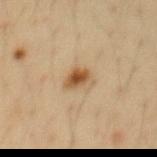Case summary:
- workup: no biopsy performed (imaged during a skin exam)
- subject: male, aged approximately 35
- automated metrics: an area of roughly 5 mm², an outline eccentricity of about 0.6 (0 = round, 1 = elongated), and a symmetry-axis asymmetry near 0.3; a lesion color around L≈49 a*≈17 b*≈34 in CIELAB, about 12 CIELAB-L* units darker than the surrounding skin, and a normalized border contrast of about 9
- site: the abdomen
- acquisition: total-body-photography crop, ~15 mm field of view
- lesion size: about 3 mm
- illumination: cross-polarized